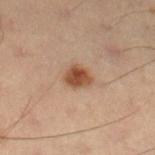notes: total-body-photography surveillance lesion; no biopsy
lesion diameter: about 3 mm
subject: male, aged 53 to 57
anatomic site: the right lower leg
image: 15 mm crop, total-body photography
lighting: cross-polarized